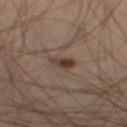Impression: The lesion was photographed on a routine skin check and not biopsied; there is no pathology result. Acquisition and patient details: From the right thigh. Longest diameter approximately 3 mm. The lesion-visualizer software estimated a mean CIELAB color near L≈29 a*≈13 b*≈20, a lesion–skin lightness drop of about 8, and a lesion-to-skin contrast of about 8.5 (normalized; higher = more distinct). The software also gave a nevus-likeness score of about 90/100 and a detector confidence of about 100 out of 100 that the crop contains a lesion. The tile uses cross-polarized illumination. A lesion tile, about 15 mm wide, cut from a 3D total-body photograph. A male patient roughly 55 years of age.